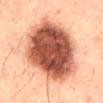Findings:
- imaging modality: ~15 mm tile from a whole-body skin photo
- diameter: about 10.5 mm
- body site: the back
- lighting: cross-polarized
- image-analysis metrics: a mean CIELAB color near L≈42 a*≈23 b*≈26; a border-irregularity index near 2/10 and a color-variation rating of about 7/10
- patient: male, aged 48 to 52